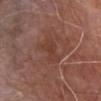follow-up — catalogued during a skin exam; not biopsied
body site — the chest
acquisition — ~15 mm crop, total-body skin-cancer survey
subject — male, aged approximately 80
illumination — white-light
diameter — ~3 mm (longest diameter)
automated lesion analysis — a footprint of about 5 mm², an outline eccentricity of about 0.75 (0 = round, 1 = elongated), and a shape-asymmetry score of about 0.45 (0 = symmetric); a border-irregularity rating of about 4.5/10 and peripheral color asymmetry of about 0.5; a classifier nevus-likeness of about 0/100 and lesion-presence confidence of about 95/100